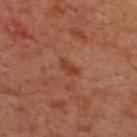Impression:
Captured during whole-body skin photography for melanoma surveillance; the lesion was not biopsied.
Image and clinical context:
A lesion tile, about 15 mm wide, cut from a 3D total-body photograph. The lesion is on the upper back. Longest diameter approximately 2.5 mm. The patient is a male aged approximately 45. The total-body-photography lesion software estimated an area of roughly 2.5 mm², an outline eccentricity of about 0.85 (0 = round, 1 = elongated), and two-axis asymmetry of about 0.45. Captured under cross-polarized illumination.